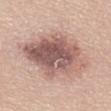The lesion was photographed on a routine skin check and not biopsied; there is no pathology result.